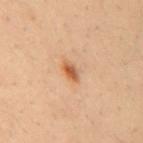workup=catalogued during a skin exam; not biopsied | lesion diameter=≈2.5 mm | patient=male, approximately 30 years of age | tile lighting=cross-polarized | body site=the mid back | imaging modality=~15 mm tile from a whole-body skin photo.